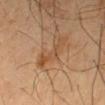The lesion was photographed on a routine skin check and not biopsied; there is no pathology result.
The lesion is located on the arm.
The subject is a male roughly 60 years of age.
The lesion-visualizer software estimated a lesion area of about 6.5 mm², an eccentricity of roughly 0.9, and two-axis asymmetry of about 0.55. It also reported a mean CIELAB color near L≈50 a*≈20 b*≈35, roughly 7 lightness units darker than nearby skin, and a normalized lesion–skin contrast near 6.
This is a cross-polarized tile.
Longest diameter approximately 4.5 mm.
This image is a 15 mm lesion crop taken from a total-body photograph.